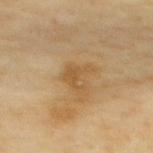follow-up: catalogued during a skin exam; not biopsied
patient: female, approximately 60 years of age
image: ~15 mm tile from a whole-body skin photo
lesion diameter: ≈3.5 mm
lighting: cross-polarized illumination
site: the back